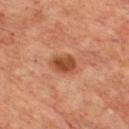Recorded during total-body skin imaging; not selected for excision or biopsy. The tile uses cross-polarized illumination. Located on the front of the torso. A roughly 15 mm field-of-view crop from a total-body skin photograph. The subject is a male in their 70s. About 3 mm across.